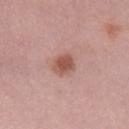Recorded during total-body skin imaging; not selected for excision or biopsy. A 15 mm close-up extracted from a 3D total-body photography capture. The lesion is located on the leg. A female patient aged around 40.